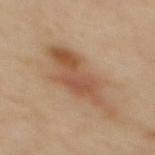Findings:
* follow-up — total-body-photography surveillance lesion; no biopsy
* size — ≈8 mm
* illumination — cross-polarized
* body site — the back
* automated lesion analysis — a lesion color around L≈54 a*≈20 b*≈33 in CIELAB, about 10 CIELAB-L* units darker than the surrounding skin, and a lesion-to-skin contrast of about 7 (normalized; higher = more distinct); a border-irregularity index near 4.5/10 and radial color variation of about 2; an automated nevus-likeness rating near 50 out of 100 and lesion-presence confidence of about 100/100
* patient — female, about 40 years old
* acquisition — total-body-photography crop, ~15 mm field of view